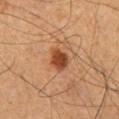The lesion was photographed on a routine skin check and not biopsied; there is no pathology result. The patient is a male aged 58–62. The recorded lesion diameter is about 3 mm. This is a cross-polarized tile. Automated image analysis of the tile measured an average lesion color of about L≈41 a*≈24 b*≈33 (CIELAB), roughly 13 lightness units darker than nearby skin, and a normalized border contrast of about 10. And it measured border irregularity of about 2 on a 0–10 scale, a within-lesion color-variation index near 3/10, and peripheral color asymmetry of about 1. From the right thigh. A 15 mm crop from a total-body photograph taken for skin-cancer surveillance.